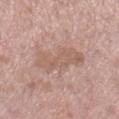Imaged during a routine full-body skin examination; the lesion was not biopsied and no histopathology is available.
Located on the right lower leg.
The subject is a female aged 48–52.
About 6.5 mm across.
Captured under white-light illumination.
A 15 mm crop from a total-body photograph taken for skin-cancer surveillance.